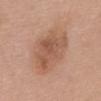Q: Was a biopsy performed?
A: catalogued during a skin exam; not biopsied
Q: Patient demographics?
A: female, aged around 60
Q: Lesion size?
A: about 8 mm
Q: What did automated image analysis measure?
A: a footprint of about 22 mm² and a shape-asymmetry score of about 0.25 (0 = symmetric); a mean CIELAB color near L≈55 a*≈21 b*≈31, about 9 CIELAB-L* units darker than the surrounding skin, and a lesion-to-skin contrast of about 6.5 (normalized; higher = more distinct); a within-lesion color-variation index near 4.5/10 and radial color variation of about 1.5; a nevus-likeness score of about 10/100 and a lesion-detection confidence of about 100/100
Q: How was the tile lit?
A: white-light
Q: What is the anatomic site?
A: the upper back
Q: What kind of image is this?
A: ~15 mm tile from a whole-body skin photo